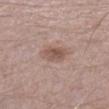Impression: Captured during whole-body skin photography for melanoma surveillance; the lesion was not biopsied. Clinical summary: Imaged with white-light lighting. A 15 mm close-up extracted from a 3D total-body photography capture. The lesion's longest dimension is about 3.5 mm. A male subject, roughly 30 years of age.